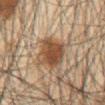Part of a total-body skin-imaging series; this lesion was reviewed on a skin check and was not flagged for biopsy. A male subject roughly 65 years of age. Located on the chest. The total-body-photography lesion software estimated a lesion–skin lightness drop of about 12. A roughly 15 mm field-of-view crop from a total-body skin photograph. Imaged with cross-polarized lighting. About 4.5 mm across.